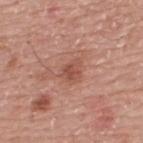Captured during whole-body skin photography for melanoma surveillance; the lesion was not biopsied. The lesion is on the back. A lesion tile, about 15 mm wide, cut from a 3D total-body photograph. The patient is a male approximately 75 years of age.